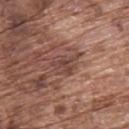The lesion was tiled from a total-body skin photograph and was not biopsied. The lesion is located on the upper back. Measured at roughly 4 mm in maximum diameter. The lesion-visualizer software estimated an area of roughly 6.5 mm² and an outline eccentricity of about 0.7 (0 = round, 1 = elongated). It also reported a within-lesion color-variation index near 3/10 and radial color variation of about 1. The software also gave an automated nevus-likeness rating near 0 out of 100 and a lesion-detection confidence of about 75/100. Captured under white-light illumination. A male subject, in their mid- to late 70s. Cropped from a whole-body photographic skin survey; the tile spans about 15 mm.